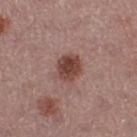follow-up: catalogued during a skin exam; not biopsied
acquisition: 15 mm crop, total-body photography
size: ~3.5 mm (longest diameter)
lighting: white-light illumination
subject: female, aged approximately 55
body site: the left thigh